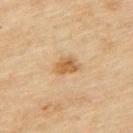Captured during whole-body skin photography for melanoma surveillance; the lesion was not biopsied.
Automated tile analysis of the lesion measured an average lesion color of about L≈50 a*≈17 b*≈36 (CIELAB), roughly 10 lightness units darker than nearby skin, and a normalized border contrast of about 8.5.
Measured at roughly 3 mm in maximum diameter.
This image is a 15 mm lesion crop taken from a total-body photograph.
The lesion is on the upper back.
A male subject, about 45 years old.
This is a cross-polarized tile.